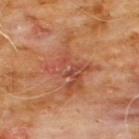notes: imaged on a skin check; not biopsied | patient: male, roughly 60 years of age | illumination: cross-polarized | body site: the chest | imaging modality: ~15 mm crop, total-body skin-cancer survey.